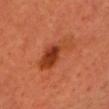Impression:
Captured during whole-body skin photography for melanoma surveillance; the lesion was not biopsied.
Image and clinical context:
Longest diameter approximately 6 mm. A female patient aged around 45. The lesion is located on the front of the torso. The tile uses cross-polarized illumination. A 15 mm close-up extracted from a 3D total-body photography capture. The total-body-photography lesion software estimated a mean CIELAB color near L≈42 a*≈32 b*≈38, roughly 11 lightness units darker than nearby skin, and a normalized border contrast of about 8. It also reported a border-irregularity rating of about 4/10, internal color variation of about 7.5 on a 0–10 scale, and radial color variation of about 2.5. The analysis additionally found an automated nevus-likeness rating near 90 out of 100 and a detector confidence of about 100 out of 100 that the crop contains a lesion.